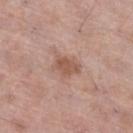Captured during whole-body skin photography for melanoma surveillance; the lesion was not biopsied. A 15 mm close-up extracted from a 3D total-body photography capture. A female subject aged 58 to 62. The total-body-photography lesion software estimated a lesion area of about 5.5 mm², a shape eccentricity near 0.7, and a shape-asymmetry score of about 0.2 (0 = symmetric). The analysis additionally found border irregularity of about 2.5 on a 0–10 scale, internal color variation of about 2 on a 0–10 scale, and a peripheral color-asymmetry measure near 1. The software also gave an automated nevus-likeness rating near 5 out of 100 and a lesion-detection confidence of about 100/100. From the right thigh.